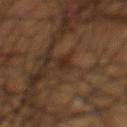Impression: Imaged during a routine full-body skin examination; the lesion was not biopsied and no histopathology is available. Clinical summary: A 15 mm close-up extracted from a 3D total-body photography capture. Captured under cross-polarized illumination. The subject is a male approximately 55 years of age. Approximately 2.5 mm at its widest. Automated tile analysis of the lesion measured roughly 6 lightness units darker than nearby skin and a normalized border contrast of about 7. The software also gave border irregularity of about 2.5 on a 0–10 scale, internal color variation of about 1 on a 0–10 scale, and radial color variation of about 0.5. Located on the front of the torso.